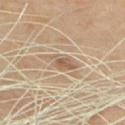{"biopsy_status": "not biopsied; imaged during a skin examination", "image": {"source": "total-body photography crop", "field_of_view_mm": 15}, "patient": {"sex": "male", "age_approx": 70}, "site": "front of the torso"}